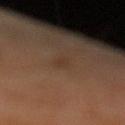Findings:
- biopsy status — imaged on a skin check; not biopsied
- automated metrics — a border-irregularity rating of about 3/10, a within-lesion color-variation index near 0/10, and radial color variation of about 0
- lesion size — ≈2.5 mm
- subject — male, aged around 60
- anatomic site — the left lower leg
- imaging modality — ~15 mm tile from a whole-body skin photo